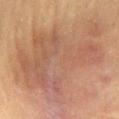Clinical impression:
This lesion was catalogued during total-body skin photography and was not selected for biopsy.
Context:
Approximately 14.5 mm at its widest. The lesion is located on the mid back. A lesion tile, about 15 mm wide, cut from a 3D total-body photograph. A male patient, about 60 years old. The lesion-visualizer software estimated a mean CIELAB color near L≈47 a*≈17 b*≈26 and a normalized lesion–skin contrast near 6.5. The analysis additionally found a border-irregularity rating of about 6.5/10 and a color-variation rating of about 5.5/10. The tile uses cross-polarized illumination.